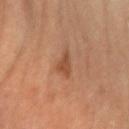Acquisition and patient details:
Captured under cross-polarized illumination. A female subject aged 63–67. The lesion is located on the left arm. Cropped from a total-body skin-imaging series; the visible field is about 15 mm. Longest diameter approximately 2.5 mm.